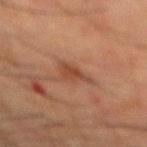Impression: The lesion was photographed on a routine skin check and not biopsied; there is no pathology result. Context: Located on the back. This image is a 15 mm lesion crop taken from a total-body photograph. Measured at roughly 3.5 mm in maximum diameter. A male patient aged approximately 65.